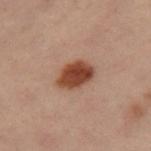Captured during whole-body skin photography for melanoma surveillance; the lesion was not biopsied. A female subject aged 58–62. The lesion-visualizer software estimated an area of roughly 9 mm², a shape eccentricity near 0.75, and two-axis asymmetry of about 0.15. And it measured a mean CIELAB color near L≈38 a*≈21 b*≈27, roughly 14 lightness units darker than nearby skin, and a normalized border contrast of about 12. The lesion is located on the left leg. The tile uses cross-polarized illumination. Measured at roughly 4 mm in maximum diameter. A close-up tile cropped from a whole-body skin photograph, about 15 mm across.